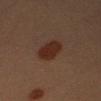This lesion was catalogued during total-body skin photography and was not selected for biopsy. The recorded lesion diameter is about 4.5 mm. A male patient, aged 38 to 42. A 15 mm close-up extracted from a 3D total-body photography capture. This is a cross-polarized tile. The lesion is on the right upper arm.